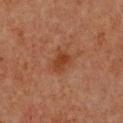Clinical impression:
No biopsy was performed on this lesion — it was imaged during a full skin examination and was not determined to be concerning.
Image and clinical context:
The lesion is on the chest. Longest diameter approximately 3 mm. Automated image analysis of the tile measured a lesion color around L≈34 a*≈23 b*≈30 in CIELAB, a lesion–skin lightness drop of about 7, and a lesion-to-skin contrast of about 7.5 (normalized; higher = more distinct). A close-up tile cropped from a whole-body skin photograph, about 15 mm across. A female patient aged around 50. This is a cross-polarized tile.